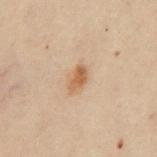<tbp_lesion>
  <biopsy_status>not biopsied; imaged during a skin examination</biopsy_status>
  <lighting>cross-polarized</lighting>
  <patient>
    <sex>female</sex>
    <age_approx>30</age_approx>
  </patient>
  <site>chest</site>
  <automated_metrics>
    <border_irregularity_0_10>2.5</border_irregularity_0_10>
    <nevus_likeness_0_100>80</nevus_likeness_0_100>
    <lesion_detection_confidence_0_100>100</lesion_detection_confidence_0_100>
  </automated_metrics>
  <image>
    <source>total-body photography crop</source>
    <field_of_view_mm>15</field_of_view_mm>
  </image>
</tbp_lesion>